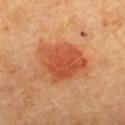Captured during whole-body skin photography for melanoma surveillance; the lesion was not biopsied.
About 5.5 mm across.
A lesion tile, about 15 mm wide, cut from a 3D total-body photograph.
The lesion is located on the upper back.
A female subject, aged 58 to 62.
The tile uses cross-polarized illumination.